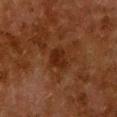Captured during whole-body skin photography for melanoma surveillance; the lesion was not biopsied.
On the chest.
A female subject about 50 years old.
Cropped from a whole-body photographic skin survey; the tile spans about 15 mm.